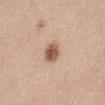Clinical impression: The lesion was photographed on a routine skin check and not biopsied; there is no pathology result. Background: Located on the abdomen. Approximately 3 mm at its widest. The tile uses white-light illumination. A close-up tile cropped from a whole-body skin photograph, about 15 mm across. Automated image analysis of the tile measured an outline eccentricity of about 0.45 (0 = round, 1 = elongated) and a symmetry-axis asymmetry near 0.2. The software also gave a lesion–skin lightness drop of about 15 and a normalized border contrast of about 9.5. The patient is a female in their mid- to late 40s.